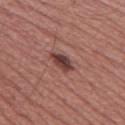Q: What lighting was used for the tile?
A: white-light
Q: What is the lesion's diameter?
A: ~3.5 mm (longest diameter)
Q: Who is the patient?
A: male, aged approximately 50
Q: How was this image acquired?
A: ~15 mm crop, total-body skin-cancer survey
Q: Where on the body is the lesion?
A: the left thigh
Q: Automated lesion metrics?
A: a footprint of about 5 mm², a shape eccentricity near 0.85, and a symmetry-axis asymmetry near 0.2; an automated nevus-likeness rating near 45 out of 100